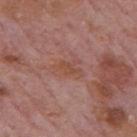| feature | finding |
|---|---|
| notes | catalogued during a skin exam; not biopsied |
| patient | male, aged 73 to 77 |
| acquisition | total-body-photography crop, ~15 mm field of view |
| diameter | ≈4 mm |
| anatomic site | the mid back |
| automated metrics | an average lesion color of about L≈49 a*≈22 b*≈27 (CIELAB), a lesion–skin lightness drop of about 5, and a normalized lesion–skin contrast near 5.5; a border-irregularity rating of about 5/10, a within-lesion color-variation index near 2.5/10, and peripheral color asymmetry of about 1; a classifier nevus-likeness of about 0/100 and a lesion-detection confidence of about 100/100 |
| illumination | white-light illumination |